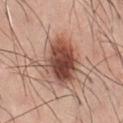| feature | finding |
|---|---|
| follow-up | imaged on a skin check; not biopsied |
| image source | 15 mm crop, total-body photography |
| body site | the chest |
| tile lighting | white-light illumination |
| patient | male, aged approximately 45 |
| size | ≈6 mm |